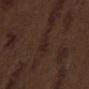Q: Is there a histopathology result?
A: imaged on a skin check; not biopsied
Q: Who is the patient?
A: male, about 70 years old
Q: Lesion location?
A: the mid back
Q: How large is the lesion?
A: ~3.5 mm (longest diameter)
Q: How was this image acquired?
A: 15 mm crop, total-body photography